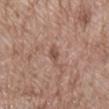Recorded during total-body skin imaging; not selected for excision or biopsy.
Cropped from a total-body skin-imaging series; the visible field is about 15 mm.
Automated tile analysis of the lesion measured a footprint of about 2.5 mm², a shape eccentricity near 0.9, and a symmetry-axis asymmetry near 0.3. And it measured an average lesion color of about L≈51 a*≈19 b*≈26 (CIELAB), a lesion–skin lightness drop of about 9, and a normalized border contrast of about 6.5. The software also gave a classifier nevus-likeness of about 0/100.
From the back.
The patient is a male aged 68 to 72.
Approximately 2.5 mm at its widest.